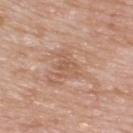<case>
<biopsy_status>not biopsied; imaged during a skin examination</biopsy_status>
<lesion_size>
  <long_diameter_mm_approx>3.5</long_diameter_mm_approx>
</lesion_size>
<image>
  <source>total-body photography crop</source>
  <field_of_view_mm>15</field_of_view_mm>
</image>
<site>upper back</site>
<lighting>white-light</lighting>
<patient>
  <sex>male</sex>
  <age_approx>60</age_approx>
</patient>
</case>